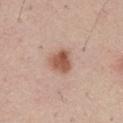| key | value |
|---|---|
| biopsy status | imaged on a skin check; not biopsied |
| image source | total-body-photography crop, ~15 mm field of view |
| body site | the chest |
| tile lighting | white-light illumination |
| patient | male, aged 58 to 62 |